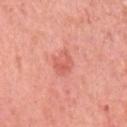- notes — imaged on a skin check; not biopsied
- tile lighting — white-light
- TBP lesion metrics — about 8 CIELAB-L* units darker than the surrounding skin and a normalized lesion–skin contrast near 5.5; a peripheral color-asymmetry measure near 1.5
- acquisition — ~15 mm crop, total-body skin-cancer survey
- patient — female, in their mid- to late 50s
- site — the left upper arm
- diameter — ≈3 mm